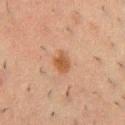Q: Was a biopsy performed?
A: imaged on a skin check; not biopsied
Q: Lesion size?
A: ~3 mm (longest diameter)
Q: Where on the body is the lesion?
A: the chest
Q: How was the tile lit?
A: cross-polarized illumination
Q: Patient demographics?
A: male, aged approximately 50
Q: What kind of image is this?
A: 15 mm crop, total-body photography